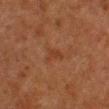follow-up — no biopsy performed (imaged during a skin exam).